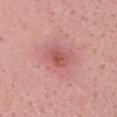| field | value |
|---|---|
| follow-up | imaged on a skin check; not biopsied |
| illumination | white-light illumination |
| diameter | ≈2.5 mm |
| location | the head or neck |
| subject | female, about 25 years old |
| image | ~15 mm tile from a whole-body skin photo |